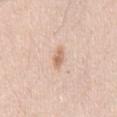A 15 mm crop from a total-body photograph taken for skin-cancer surveillance. The subject is a male aged 33–37. Located on the mid back.